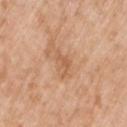The lesion was tiled from a total-body skin photograph and was not biopsied.
A 15 mm close-up tile from a total-body photography series done for melanoma screening.
On the left upper arm.
Captured under white-light illumination.
The subject is a male approximately 50 years of age.
Approximately 3 mm at its widest.